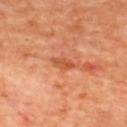Recorded during total-body skin imaging; not selected for excision or biopsy. From the upper back. Imaged with cross-polarized lighting. A male subject, about 60 years old. Automated tile analysis of the lesion measured an area of roughly 3.5 mm², a shape eccentricity near 0.85, and a symmetry-axis asymmetry near 0.35. The software also gave a lesion color around L≈54 a*≈30 b*≈39 in CIELAB and a lesion-to-skin contrast of about 6.5 (normalized; higher = more distinct). This image is a 15 mm lesion crop taken from a total-body photograph. Approximately 3 mm at its widest.